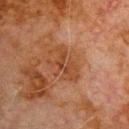Recorded during total-body skin imaging; not selected for excision or biopsy. A region of skin cropped from a whole-body photographic capture, roughly 15 mm wide. A male patient roughly 80 years of age. The lesion is located on the chest.